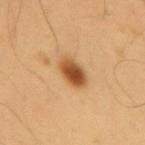Recorded during total-body skin imaging; not selected for excision or biopsy. On the mid back. Imaged with cross-polarized lighting. Cropped from a total-body skin-imaging series; the visible field is about 15 mm. A male subject, in their mid- to late 50s. Longest diameter approximately 4 mm. The total-body-photography lesion software estimated a lesion area of about 7.5 mm², an eccentricity of roughly 0.8, and a symmetry-axis asymmetry near 0.2. The software also gave border irregularity of about 1.5 on a 0–10 scale, a within-lesion color-variation index near 6/10, and peripheral color asymmetry of about 2. It also reported an automated nevus-likeness rating near 100 out of 100.